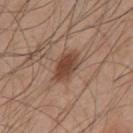biopsy status — imaged on a skin check; not biopsied | diameter — about 4 mm | anatomic site — the chest | tile lighting — white-light illumination | imaging modality — total-body-photography crop, ~15 mm field of view | automated lesion analysis — a border-irregularity index near 2/10 and a peripheral color-asymmetry measure near 1; a classifier nevus-likeness of about 90/100 and lesion-presence confidence of about 100/100 | patient — male, aged around 45.